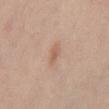Q: Is there a histopathology result?
A: total-body-photography surveillance lesion; no biopsy
Q: What is the lesion's diameter?
A: ≈2.5 mm
Q: What is the anatomic site?
A: the abdomen
Q: What did automated image analysis measure?
A: a lesion color around L≈60 a*≈19 b*≈31 in CIELAB and a lesion-to-skin contrast of about 6 (normalized; higher = more distinct); lesion-presence confidence of about 100/100
Q: What kind of image is this?
A: total-body-photography crop, ~15 mm field of view
Q: Who is the patient?
A: male, aged 33–37
Q: How was the tile lit?
A: white-light